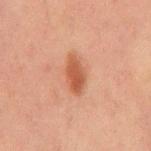Part of a total-body skin-imaging series; this lesion was reviewed on a skin check and was not flagged for biopsy.
The lesion is located on the abdomen.
The recorded lesion diameter is about 4 mm.
A 15 mm close-up tile from a total-body photography series done for melanoma screening.
A male subject, in their mid- to late 60s.
Automated image analysis of the tile measured an area of roughly 6 mm², an eccentricity of roughly 0.9, and a symmetry-axis asymmetry near 0.15. It also reported about 10 CIELAB-L* units darker than the surrounding skin and a lesion-to-skin contrast of about 8 (normalized; higher = more distinct). And it measured a border-irregularity rating of about 2/10 and a color-variation rating of about 2/10.